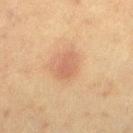  image:
    source: total-body photography crop
    field_of_view_mm: 15
  patient:
    sex: female
    age_approx: 50
  lighting: cross-polarized
  site: left lower leg
  lesion_size:
    long_diameter_mm_approx: 3.0
  automated_metrics:
    area_mm2_approx: 5.0
    shape_asymmetry: 0.2